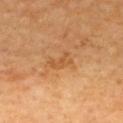This is a cross-polarized tile. On the upper back. A 15 mm close-up extracted from a 3D total-body photography capture. A female patient. The lesion's longest dimension is about 3 mm. Automated image analysis of the tile measured an eccentricity of roughly 0.85. The software also gave internal color variation of about 0.5 on a 0–10 scale and radial color variation of about 0. The software also gave a nevus-likeness score of about 0/100 and lesion-presence confidence of about 100/100.